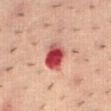Q: Was a biopsy performed?
A: catalogued during a skin exam; not biopsied
Q: Lesion size?
A: ~4 mm (longest diameter)
Q: What are the patient's age and sex?
A: male, aged approximately 55
Q: What is the anatomic site?
A: the mid back
Q: What did automated image analysis measure?
A: a lesion area of about 9.5 mm², an outline eccentricity of about 0.65 (0 = round, 1 = elongated), and two-axis asymmetry of about 0.2; roughly 16 lightness units darker than nearby skin and a normalized border contrast of about 12; border irregularity of about 1.5 on a 0–10 scale
Q: What is the imaging modality?
A: ~15 mm tile from a whole-body skin photo